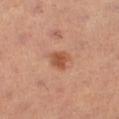<lesion>
<biopsy_status>not biopsied; imaged during a skin examination</biopsy_status>
</lesion>